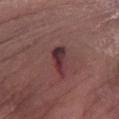follow-up=imaged on a skin check; not biopsied
illumination=white-light
imaging modality=15 mm crop, total-body photography
site=the arm
subject=male, aged 78–82